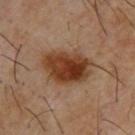biopsy status: imaged on a skin check; not biopsied
acquisition: ~15 mm crop, total-body skin-cancer survey
illumination: cross-polarized
patient: male, roughly 65 years of age
location: the upper back
lesion diameter: ≈6 mm